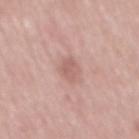notes = imaged on a skin check; not biopsied
imaging modality = total-body-photography crop, ~15 mm field of view
site = the mid back
lesion diameter = ≈3 mm
automated lesion analysis = an area of roughly 4 mm² and two-axis asymmetry of about 0.3; a mean CIELAB color near L≈60 a*≈21 b*≈24 and a normalized lesion–skin contrast near 5.5; a border-irregularity index near 3/10, a within-lesion color-variation index near 1.5/10, and radial color variation of about 0.5
subject = male, approximately 50 years of age